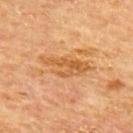This lesion was catalogued during total-body skin photography and was not selected for biopsy. The tile uses cross-polarized illumination. The recorded lesion diameter is about 6 mm. The lesion is on the mid back. Cropped from a total-body skin-imaging series; the visible field is about 15 mm. A male patient, roughly 65 years of age.